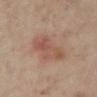The lesion was photographed on a routine skin check and not biopsied; there is no pathology result. From the right lower leg. Measured at roughly 6 mm in maximum diameter. A 15 mm close-up tile from a total-body photography series done for melanoma screening. The lesion-visualizer software estimated an average lesion color of about L≈50 a*≈20 b*≈26 (CIELAB) and a lesion-to-skin contrast of about 6 (normalized; higher = more distinct). And it measured a border-irregularity rating of about 4.5/10, a within-lesion color-variation index near 5/10, and a peripheral color-asymmetry measure near 1.5. The subject is a female aged 33–37. This is a cross-polarized tile.